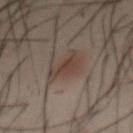Part of a total-body skin-imaging series; this lesion was reviewed on a skin check and was not flagged for biopsy. The lesion's longest dimension is about 4 mm. An algorithmic analysis of the crop reported a mean CIELAB color near L≈39 a*≈14 b*≈22, about 7 CIELAB-L* units darker than the surrounding skin, and a normalized lesion–skin contrast near 7. It also reported a border-irregularity rating of about 4.5/10. It also reported an automated nevus-likeness rating near 100 out of 100 and a lesion-detection confidence of about 100/100. A male patient aged 38–42. A close-up tile cropped from a whole-body skin photograph, about 15 mm across. The lesion is located on the front of the torso. Imaged with cross-polarized lighting.